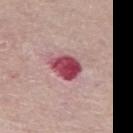biopsy_status: not biopsied; imaged during a skin examination
lesion_size:
  long_diameter_mm_approx: 4.0
patient:
  sex: female
  age_approx: 65
image:
  source: total-body photography crop
  field_of_view_mm: 15
site: left thigh
automated_metrics:
  area_mm2_approx: 9.0
  eccentricity: 0.7
  shape_asymmetry: 0.2
  cielab_L: 48
  cielab_a: 35
  cielab_b: 17
  vs_skin_darker_L: 18.0
  vs_skin_contrast_norm: 12.5
  border_irregularity_0_10: 2.0
  color_variation_0_10: 5.5
  peripheral_color_asymmetry: 2.0